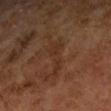{
  "biopsy_status": "not biopsied; imaged during a skin examination",
  "site": "left forearm",
  "patient": {
    "sex": "female",
    "age_approx": 60
  },
  "lesion_size": {
    "long_diameter_mm_approx": 5.0
  },
  "image": {
    "source": "total-body photography crop",
    "field_of_view_mm": 15
  },
  "lighting": "cross-polarized"
}